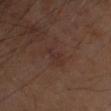Case summary:
- follow-up · no biopsy performed (imaged during a skin exam)
- automated metrics · an outline eccentricity of about 0.9 (0 = round, 1 = elongated) and a shape-asymmetry score of about 0.4 (0 = symmetric); a lesion–skin lightness drop of about 4 and a normalized border contrast of about 4.5; a border-irregularity index near 5/10, internal color variation of about 0 on a 0–10 scale, and radial color variation of about 0
- image · 15 mm crop, total-body photography
- lesion diameter · ~3 mm (longest diameter)
- lighting · cross-polarized illumination
- patient · male, aged approximately 70
- anatomic site · the left forearm